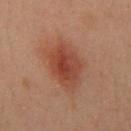Recorded during total-body skin imaging; not selected for excision or biopsy. About 6.5 mm across. The lesion-visualizer software estimated an area of roughly 19 mm² and a shape eccentricity near 0.75. The software also gave a border-irregularity rating of about 2.5/10, a within-lesion color-variation index near 4/10, and radial color variation of about 1. A 15 mm close-up tile from a total-body photography series done for melanoma screening. On the chest. A male patient aged 58–62. Imaged with cross-polarized lighting.